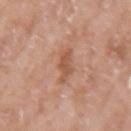site: right forearm
lesion_size:
  long_diameter_mm_approx: 3.5
patient:
  sex: female
  age_approx: 75
automated_metrics:
  area_mm2_approx: 5.0
  eccentricity: 0.8
  shape_asymmetry: 0.35
  nevus_likeness_0_100: 0
  lesion_detection_confidence_0_100: 100
image:
  source: total-body photography crop
  field_of_view_mm: 15
lighting: white-light